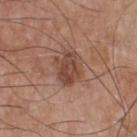This lesion was catalogued during total-body skin photography and was not selected for biopsy. The lesion is located on the chest. A lesion tile, about 15 mm wide, cut from a 3D total-body photograph. A male subject approximately 65 years of age. The tile uses white-light illumination.